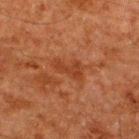Captured during whole-body skin photography for melanoma surveillance; the lesion was not biopsied. A 15 mm close-up extracted from a 3D total-body photography capture. Measured at roughly 3.5 mm in maximum diameter. The tile uses cross-polarized illumination. On the upper back. The patient is a male approximately 60 years of age.